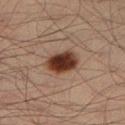Q: Is there a histopathology result?
A: catalogued during a skin exam; not biopsied
Q: What is the imaging modality?
A: 15 mm crop, total-body photography
Q: Who is the patient?
A: male, in their mid-30s
Q: Automated lesion metrics?
A: an average lesion color of about L≈32 a*≈20 b*≈25 (CIELAB) and a normalized border contrast of about 14.5; a border-irregularity rating of about 2/10, internal color variation of about 5 on a 0–10 scale, and radial color variation of about 1.5
Q: What lighting was used for the tile?
A: cross-polarized illumination
Q: Where on the body is the lesion?
A: the left lower leg
Q: How large is the lesion?
A: ~4 mm (longest diameter)